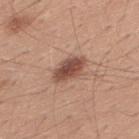| field | value |
|---|---|
| lesion diameter | ~4.5 mm (longest diameter) |
| subject | male, in their 30s |
| image source | total-body-photography crop, ~15 mm field of view |
| body site | the back |